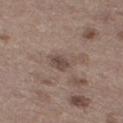Assessment:
No biopsy was performed on this lesion — it was imaged during a full skin examination and was not determined to be concerning.
Image and clinical context:
The patient is a male about 70 years old. This is a white-light tile. On the left lower leg. A 15 mm close-up extracted from a 3D total-body photography capture.